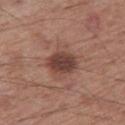Q: Is there a histopathology result?
A: catalogued during a skin exam; not biopsied
Q: How large is the lesion?
A: ~3.5 mm (longest diameter)
Q: Lesion location?
A: the right thigh
Q: What did automated image analysis measure?
A: an automated nevus-likeness rating near 50 out of 100 and lesion-presence confidence of about 100/100
Q: What are the patient's age and sex?
A: male, in their mid-50s
Q: How was the tile lit?
A: white-light
Q: How was this image acquired?
A: 15 mm crop, total-body photography